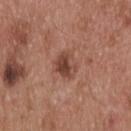This lesion was catalogued during total-body skin photography and was not selected for biopsy. The lesion-visualizer software estimated a lesion–skin lightness drop of about 11. A 15 mm close-up extracted from a 3D total-body photography capture. Approximately 3 mm at its widest. This is a white-light tile. A male subject approximately 55 years of age. The lesion is located on the upper back.